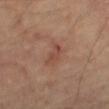{
  "biopsy_status": "not biopsied; imaged during a skin examination",
  "lighting": "cross-polarized",
  "image": {
    "source": "total-body photography crop",
    "field_of_view_mm": 15
  },
  "patient": {
    "sex": "male",
    "age_approx": 70
  },
  "site": "leg"
}